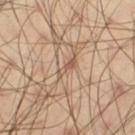biopsy_status: not biopsied; imaged during a skin examination
patient:
  sex: male
  age_approx: 40
image:
  source: total-body photography crop
  field_of_view_mm: 15
site: left thigh
automated_metrics:
  cielab_L: 56
  cielab_a: 18
  cielab_b: 29
  vs_skin_darker_L: 9.0
  vs_skin_contrast_norm: 6.0
  color_variation_0_10: 0.0
  peripheral_color_asymmetry: 0.0
  nevus_likeness_0_100: 0
  lesion_detection_confidence_0_100: 90
lesion_size:
  long_diameter_mm_approx: 3.0